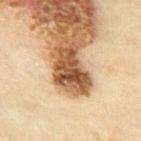* notes — no biopsy performed (imaged during a skin exam)
* lighting — cross-polarized
* size — ~5.5 mm (longest diameter)
* acquisition — ~15 mm crop, total-body skin-cancer survey
* anatomic site — the abdomen
* subject — male, roughly 70 years of age